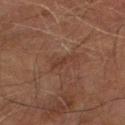{"lesion_size": {"long_diameter_mm_approx": 2.5}, "image": {"source": "total-body photography crop", "field_of_view_mm": 15}, "patient": {"sex": "male", "age_approx": 75}, "site": "right thigh"}